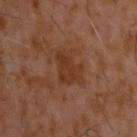The lesion was photographed on a routine skin check and not biopsied; there is no pathology result.
The tile uses cross-polarized illumination.
Automated tile analysis of the lesion measured a footprint of about 9.5 mm², a shape eccentricity near 0.65, and a shape-asymmetry score of about 0.35 (0 = symmetric). And it measured a lesion color around L≈32 a*≈21 b*≈28 in CIELAB, about 6 CIELAB-L* units darker than the surrounding skin, and a lesion-to-skin contrast of about 6.5 (normalized; higher = more distinct). It also reported a nevus-likeness score of about 0/100 and a lesion-detection confidence of about 100/100.
This image is a 15 mm lesion crop taken from a total-body photograph.
Located on the upper back.
Measured at roughly 3.5 mm in maximum diameter.
The patient is a male roughly 60 years of age.